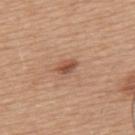The lesion was tiled from a total-body skin photograph and was not biopsied. Cropped from a total-body skin-imaging series; the visible field is about 15 mm. Located on the upper back. Imaged with white-light lighting. An algorithmic analysis of the crop reported a border-irregularity rating of about 2/10, internal color variation of about 3 on a 0–10 scale, and a peripheral color-asymmetry measure near 1. The subject is a male about 65 years old. Measured at roughly 2.5 mm in maximum diameter.